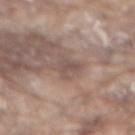<record>
<biopsy_status>not biopsied; imaged during a skin examination</biopsy_status>
<patient>
  <sex>male</sex>
  <age_approx>80</age_approx>
</patient>
<lesion_size>
  <long_diameter_mm_approx>3.0</long_diameter_mm_approx>
</lesion_size>
<lighting>white-light</lighting>
<site>mid back</site>
<image>
  <source>total-body photography crop</source>
  <field_of_view_mm>15</field_of_view_mm>
</image>
<automated_metrics>
  <area_mm2_approx>4.0</area_mm2_approx>
  <eccentricity>0.6</eccentricity>
  <shape_asymmetry>0.45</shape_asymmetry>
</automated_metrics>
</record>